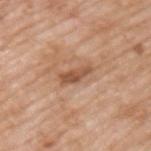Case summary:
• follow-up: catalogued during a skin exam; not biopsied
• patient: male, roughly 60 years of age
• image: 15 mm crop, total-body photography
• lesion diameter: ~3.5 mm (longest diameter)
• site: the upper back